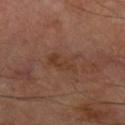Assessment: Recorded during total-body skin imaging; not selected for excision or biopsy. Context: Captured under cross-polarized illumination. Located on the leg. The subject is a male in their 70s. Cropped from a total-body skin-imaging series; the visible field is about 15 mm. The total-body-photography lesion software estimated border irregularity of about 6 on a 0–10 scale and internal color variation of about 2.5 on a 0–10 scale. The software also gave a lesion-detection confidence of about 100/100.